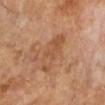The patient is a female in their mid-60s. This is a cross-polarized tile. From the right lower leg. This image is a 15 mm lesion crop taken from a total-body photograph.